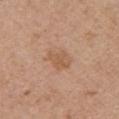notes=no biopsy performed (imaged during a skin exam); lesion size=about 3.5 mm; subject=female, in their 60s; illumination=white-light illumination; site=the arm; image=15 mm crop, total-body photography.